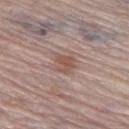The subject is a male aged around 80.
This is a white-light tile.
From the left thigh.
An algorithmic analysis of the crop reported a shape-asymmetry score of about 0.25 (0 = symmetric).
Measured at roughly 3 mm in maximum diameter.
Cropped from a total-body skin-imaging series; the visible field is about 15 mm.